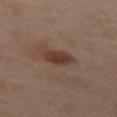Part of a total-body skin-imaging series; this lesion was reviewed on a skin check and was not flagged for biopsy.
The total-body-photography lesion software estimated a footprint of about 6.5 mm², an outline eccentricity of about 0.8 (0 = round, 1 = elongated), and two-axis asymmetry of about 0.25. It also reported a border-irregularity index near 2.5/10 and internal color variation of about 2.5 on a 0–10 scale.
Captured under cross-polarized illumination.
The lesion is on the left thigh.
Approximately 4 mm at its widest.
A region of skin cropped from a whole-body photographic capture, roughly 15 mm wide.
The patient is a female aged 48 to 52.